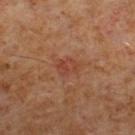Clinical impression:
The lesion was tiled from a total-body skin photograph and was not biopsied.
Context:
A male subject roughly 60 years of age. Located on the right lower leg. Cropped from a whole-body photographic skin survey; the tile spans about 15 mm.